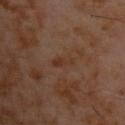Captured during whole-body skin photography for melanoma surveillance; the lesion was not biopsied. A male subject, aged around 60. A close-up tile cropped from a whole-body skin photograph, about 15 mm across. This is a cross-polarized tile. The total-body-photography lesion software estimated an area of roughly 3 mm². The software also gave a lesion color around L≈31 a*≈17 b*≈25 in CIELAB, a lesion–skin lightness drop of about 5, and a normalized lesion–skin contrast near 5.5. The lesion is located on the back.